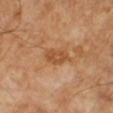{"biopsy_status": "not biopsied; imaged during a skin examination", "image": {"source": "total-body photography crop", "field_of_view_mm": 15}, "patient": {"sex": "male", "age_approx": 65}}